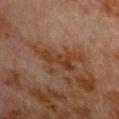follow-up: total-body-photography surveillance lesion; no biopsy
site: the chest
automated metrics: an eccentricity of roughly 0.9 and two-axis asymmetry of about 0.4; an average lesion color of about L≈29 a*≈17 b*≈25 (CIELAB), about 6 CIELAB-L* units darker than the surrounding skin, and a lesion-to-skin contrast of about 8 (normalized; higher = more distinct); a border-irregularity index near 6/10 and radial color variation of about 0.5; a classifier nevus-likeness of about 0/100 and a lesion-detection confidence of about 100/100
subject: male, approximately 80 years of age
size: ≈5 mm
tile lighting: cross-polarized
acquisition: 15 mm crop, total-body photography